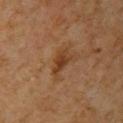<tbp_lesion>
  <biopsy_status>not biopsied; imaged during a skin examination</biopsy_status>
  <lighting>cross-polarized</lighting>
  <automated_metrics>
    <area_mm2_approx>5.0</area_mm2_approx>
    <eccentricity>0.8</eccentricity>
    <shape_asymmetry>0.4</shape_asymmetry>
    <cielab_L>35</cielab_L>
    <cielab_a>20</cielab_a>
    <cielab_b>31</cielab_b>
    <vs_skin_darker_L>8.0</vs_skin_darker_L>
    <nevus_likeness_0_100>20</nevus_likeness_0_100>
    <lesion_detection_confidence_0_100>100</lesion_detection_confidence_0_100>
  </automated_metrics>
  <lesion_size>
    <long_diameter_mm_approx>3.0</long_diameter_mm_approx>
  </lesion_size>
  <site>left upper arm</site>
  <patient>
    <sex>male</sex>
    <age_approx>60</age_approx>
  </patient>
  <image>
    <source>total-body photography crop</source>
    <field_of_view_mm>15</field_of_view_mm>
  </image>
</tbp_lesion>